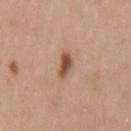Part of a total-body skin-imaging series; this lesion was reviewed on a skin check and was not flagged for biopsy. An algorithmic analysis of the crop reported an average lesion color of about L≈51 a*≈21 b*≈29 (CIELAB), roughly 14 lightness units darker than nearby skin, and a lesion-to-skin contrast of about 9.5 (normalized; higher = more distinct). The analysis additionally found peripheral color asymmetry of about 2. The software also gave a lesion-detection confidence of about 100/100. The recorded lesion diameter is about 3 mm. Captured under white-light illumination. A 15 mm crop from a total-body photograph taken for skin-cancer surveillance. A male patient, roughly 35 years of age. The lesion is located on the mid back.